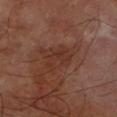<record>
  <biopsy_status>not biopsied; imaged during a skin examination</biopsy_status>
  <site>right forearm</site>
  <lighting>cross-polarized</lighting>
  <lesion_size>
    <long_diameter_mm_approx>4.0</long_diameter_mm_approx>
  </lesion_size>
  <automated_metrics>
    <area_mm2_approx>7.5</area_mm2_approx>
    <eccentricity>0.75</eccentricity>
    <shape_asymmetry>0.3</shape_asymmetry>
    <cielab_L>32</cielab_L>
    <cielab_a>21</cielab_a>
    <cielab_b>25</cielab_b>
    <vs_skin_darker_L>5.0</vs_skin_darker_L>
    <border_irregularity_0_10>3.0</border_irregularity_0_10>
    <color_variation_0_10>3.0</color_variation_0_10>
  </automated_metrics>
  <patient>
    <sex>male</sex>
    <age_approx>70</age_approx>
  </patient>
  <image>
    <source>total-body photography crop</source>
    <field_of_view_mm>15</field_of_view_mm>
  </image>
</record>